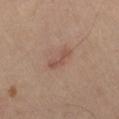Clinical impression:
The lesion was tiled from a total-body skin photograph and was not biopsied.
Acquisition and patient details:
Located on the right thigh. A male subject, roughly 65 years of age. A region of skin cropped from a whole-body photographic capture, roughly 15 mm wide.